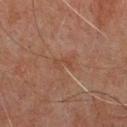Impression: The lesion was photographed on a routine skin check and not biopsied; there is no pathology result. Acquisition and patient details: The lesion's longest dimension is about 2.5 mm. Automated image analysis of the tile measured a lesion area of about 3 mm². It also reported internal color variation of about 0 on a 0–10 scale and peripheral color asymmetry of about 0. The software also gave an automated nevus-likeness rating near 0 out of 100. A 15 mm close-up tile from a total-body photography series done for melanoma screening. The patient is a male aged around 60. The lesion is on the chest.